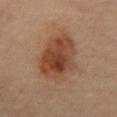imaging modality = 15 mm crop, total-body photography
tile lighting = cross-polarized
lesion diameter = ~7.5 mm (longest diameter)
body site = the chest